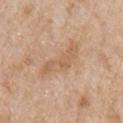Q: Was this lesion biopsied?
A: catalogued during a skin exam; not biopsied
Q: What lighting was used for the tile?
A: white-light
Q: Who is the patient?
A: male, aged 63 to 67
Q: How was this image acquired?
A: ~15 mm crop, total-body skin-cancer survey
Q: What is the anatomic site?
A: the arm
Q: Lesion size?
A: ~6 mm (longest diameter)